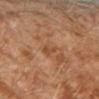Notes:
• workup: imaged on a skin check; not biopsied
• body site: the left lower leg
• patient: male, about 30 years old
• diameter: ≈2.5 mm
• lighting: cross-polarized illumination
• TBP lesion metrics: a mean CIELAB color near L≈47 a*≈22 b*≈34 and a lesion–skin lightness drop of about 7; border irregularity of about 5 on a 0–10 scale, internal color variation of about 0 on a 0–10 scale, and peripheral color asymmetry of about 0; an automated nevus-likeness rating near 0 out of 100 and a lesion-detection confidence of about 100/100
• imaging modality: total-body-photography crop, ~15 mm field of view